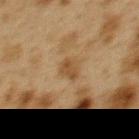• body site: the back
• acquisition: ~15 mm crop, total-body skin-cancer survey
• lesion size: ~2.5 mm (longest diameter)
• image-analysis metrics: lesion-presence confidence of about 100/100
• tile lighting: cross-polarized illumination
• patient: female, aged around 40